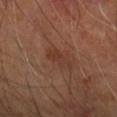biopsy status: no biopsy performed (imaged during a skin exam) | illumination: cross-polarized illumination | diameter: ≈3 mm | automated metrics: an average lesion color of about L≈34 a*≈21 b*≈27 (CIELAB) and a lesion-to-skin contrast of about 5.5 (normalized; higher = more distinct); border irregularity of about 4.5 on a 0–10 scale, a color-variation rating of about 2/10, and peripheral color asymmetry of about 0.5; a classifier nevus-likeness of about 0/100 and a detector confidence of about 100 out of 100 that the crop contains a lesion | anatomic site: the right forearm | image: ~15 mm tile from a whole-body skin photo | patient: male, approximately 70 years of age.